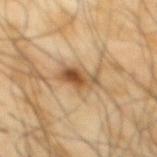biopsy status: no biopsy performed (imaged during a skin exam) | tile lighting: cross-polarized | lesion diameter: ≈4 mm | body site: the mid back | subject: male, roughly 65 years of age | image source: ~15 mm tile from a whole-body skin photo | automated lesion analysis: an area of roughly 7.5 mm², a shape eccentricity near 0.8, and a shape-asymmetry score of about 0.3 (0 = symmetric); a lesion–skin lightness drop of about 12 and a normalized lesion–skin contrast near 9; a border-irregularity rating of about 4/10, internal color variation of about 9 on a 0–10 scale, and radial color variation of about 3.5.